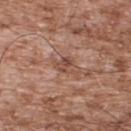Assessment: Part of a total-body skin-imaging series; this lesion was reviewed on a skin check and was not flagged for biopsy. Context: Approximately 3 mm at its widest. The lesion is on the upper back. Imaged with white-light lighting. Cropped from a whole-body photographic skin survey; the tile spans about 15 mm. The patient is a male approximately 55 years of age.